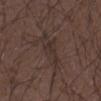Q: Is there a histopathology result?
A: imaged on a skin check; not biopsied
Q: What is the imaging modality?
A: total-body-photography crop, ~15 mm field of view
Q: Who is the patient?
A: male, approximately 50 years of age
Q: What is the anatomic site?
A: the right forearm
Q: How was the tile lit?
A: white-light